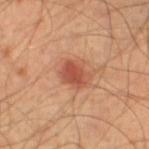Q: What are the patient's age and sex?
A: male, aged around 55
Q: How was this image acquired?
A: ~15 mm tile from a whole-body skin photo
Q: Automated lesion metrics?
A: an area of roughly 6.5 mm², an eccentricity of roughly 0.65, and two-axis asymmetry of about 0.2
Q: How large is the lesion?
A: about 3.5 mm
Q: Lesion location?
A: the left thigh